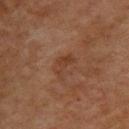Acquisition and patient details: A female subject, aged 68 to 72. The lesion-visualizer software estimated an outline eccentricity of about 0.85 (0 = round, 1 = elongated) and a shape-asymmetry score of about 0.6 (0 = symmetric). The analysis additionally found a detector confidence of about 100 out of 100 that the crop contains a lesion. The lesion is located on the back. A 15 mm close-up extracted from a 3D total-body photography capture. Imaged with cross-polarized lighting. About 3.5 mm across.